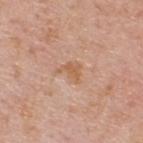Part of a total-body skin-imaging series; this lesion was reviewed on a skin check and was not flagged for biopsy. This is a white-light tile. Cropped from a whole-body photographic skin survey; the tile spans about 15 mm. A male subject, aged around 75. Located on the upper back.